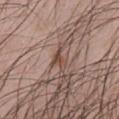The lesion was tiled from a total-body skin photograph and was not biopsied. This is a white-light tile. A male patient, aged approximately 25. On the chest. Approximately 3 mm at its widest. The total-body-photography lesion software estimated an average lesion color of about L≈47 a*≈17 b*≈24 (CIELAB), about 10 CIELAB-L* units darker than the surrounding skin, and a normalized lesion–skin contrast near 7.5. The software also gave a nevus-likeness score of about 0/100. Cropped from a total-body skin-imaging series; the visible field is about 15 mm.